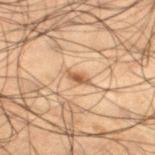Q: Is there a histopathology result?
A: imaged on a skin check; not biopsied
Q: Patient demographics?
A: male, roughly 50 years of age
Q: Lesion location?
A: the left lower leg
Q: How was this image acquired?
A: 15 mm crop, total-body photography